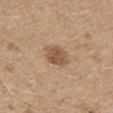Clinical impression: This lesion was catalogued during total-body skin photography and was not selected for biopsy. Context: Measured at roughly 3 mm in maximum diameter. This is a white-light tile. This image is a 15 mm lesion crop taken from a total-body photograph. A male subject, about 50 years old. The lesion is located on the left upper arm.